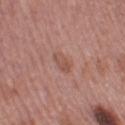Captured during whole-body skin photography for melanoma surveillance; the lesion was not biopsied.
The tile uses white-light illumination.
From the left thigh.
A 15 mm crop from a total-body photograph taken for skin-cancer surveillance.
The recorded lesion diameter is about 3 mm.
Automated tile analysis of the lesion measured an outline eccentricity of about 0.75 (0 = round, 1 = elongated) and a shape-asymmetry score of about 0.2 (0 = symmetric). And it measured a mean CIELAB color near L≈52 a*≈22 b*≈26, roughly 7 lightness units darker than nearby skin, and a normalized border contrast of about 5. The software also gave a border-irregularity index near 2/10, a color-variation rating of about 2.5/10, and peripheral color asymmetry of about 1. The software also gave an automated nevus-likeness rating near 10 out of 100 and a detector confidence of about 100 out of 100 that the crop contains a lesion.
A female patient, about 50 years old.